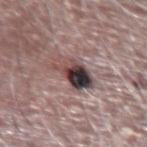  biopsy_status: not biopsied; imaged during a skin examination
  image:
    source: total-body photography crop
    field_of_view_mm: 15
  lesion_size:
    long_diameter_mm_approx: 4.5
  lighting: white-light
  site: right forearm
  patient:
    sex: male
    age_approx: 65
  automated_metrics:
    area_mm2_approx: 10.0
    eccentricity: 0.75
    shape_asymmetry: 0.3
    border_irregularity_0_10: 3.5
    peripheral_color_asymmetry: 7.5
    lesion_detection_confidence_0_100: 90